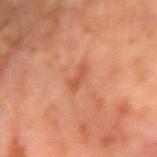follow-up: total-body-photography surveillance lesion; no biopsy
automated metrics: an average lesion color of about L≈51 a*≈28 b*≈34 (CIELAB), a lesion–skin lightness drop of about 8, and a lesion-to-skin contrast of about 6 (normalized; higher = more distinct); border irregularity of about 4.5 on a 0–10 scale and internal color variation of about 0 on a 0–10 scale
illumination: cross-polarized
diameter: ≈2.5 mm
image: ~15 mm crop, total-body skin-cancer survey
patient: male, aged approximately 60
site: the right upper arm